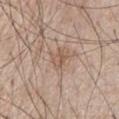Imaged during a routine full-body skin examination; the lesion was not biopsied and no histopathology is available. Approximately 2.5 mm at its widest. A male patient aged approximately 65. From the chest. Captured under white-light illumination. This image is a 15 mm lesion crop taken from a total-body photograph.